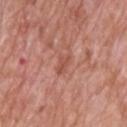Background:
Automated tile analysis of the lesion measured a lesion–skin lightness drop of about 8. The software also gave a border-irregularity index near 4/10, a color-variation rating of about 1/10, and radial color variation of about 0.5. The software also gave a classifier nevus-likeness of about 0/100 and a lesion-detection confidence of about 100/100. A male subject aged approximately 70. This is a white-light tile. About 2.5 mm across. A 15 mm crop from a total-body photograph taken for skin-cancer surveillance. From the upper back.